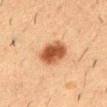Assessment: Imaged during a routine full-body skin examination; the lesion was not biopsied and no histopathology is available. Image and clinical context: About 4.5 mm across. A male subject aged around 55. Automated tile analysis of the lesion measured a lesion color around L≈48 a*≈23 b*≈35 in CIELAB and about 16 CIELAB-L* units darker than the surrounding skin. From the front of the torso. A lesion tile, about 15 mm wide, cut from a 3D total-body photograph.